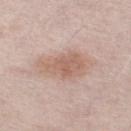| key | value |
|---|---|
| workup | total-body-photography surveillance lesion; no biopsy |
| patient | male, aged around 60 |
| location | the right thigh |
| diameter | ~6 mm (longest diameter) |
| imaging modality | ~15 mm crop, total-body skin-cancer survey |
| lighting | white-light illumination |
| automated lesion analysis | a detector confidence of about 100 out of 100 that the crop contains a lesion |